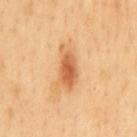The lesion was photographed on a routine skin check and not biopsied; there is no pathology result. About 5 mm across. A 15 mm close-up tile from a total-body photography series done for melanoma screening. The lesion is on the back. An algorithmic analysis of the crop reported two-axis asymmetry of about 0.25. The analysis additionally found an average lesion color of about L≈62 a*≈25 b*≈42 (CIELAB) and about 13 CIELAB-L* units darker than the surrounding skin. The analysis additionally found a nevus-likeness score of about 100/100. Captured under cross-polarized illumination. A male patient about 50 years old.